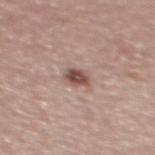<case>
<biopsy_status>not biopsied; imaged during a skin examination</biopsy_status>
<patient>
  <sex>female</sex>
  <age_approx>50</age_approx>
</patient>
<automated_metrics>
  <area_mm2_approx>4.0</area_mm2_approx>
  <eccentricity>0.65</eccentricity>
  <shape_asymmetry>0.25</shape_asymmetry>
  <vs_skin_darker_L>14.0</vs_skin_darker_L>
  <vs_skin_contrast_norm>10.0</vs_skin_contrast_norm>
  <border_irregularity_0_10>2.0</border_irregularity_0_10>
  <nevus_likeness_0_100>95</nevus_likeness_0_100>
</automated_metrics>
<site>upper back</site>
<image>
  <source>total-body photography crop</source>
  <field_of_view_mm>15</field_of_view_mm>
</image>
</case>